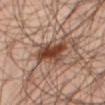Assessment: Imaged during a routine full-body skin examination; the lesion was not biopsied and no histopathology is available. Clinical summary: A 15 mm close-up tile from a total-body photography series done for melanoma screening. The lesion is on the leg. The subject is a male aged approximately 45.